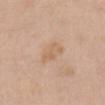{"biopsy_status": "not biopsied; imaged during a skin examination", "lighting": "white-light", "automated_metrics": {"shape_asymmetry": 0.45, "cielab_L": 63, "cielab_a": 17, "cielab_b": 33, "vs_skin_contrast_norm": 5.0, "border_irregularity_0_10": 6.5, "color_variation_0_10": 0.0, "peripheral_color_asymmetry": 0.0}, "site": "front of the torso", "image": {"source": "total-body photography crop", "field_of_view_mm": 15}, "patient": {"sex": "female", "age_approx": 70}, "lesion_size": {"long_diameter_mm_approx": 3.5}}